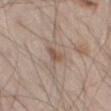Case summary:
- biopsy status — imaged on a skin check; not biopsied
- acquisition — 15 mm crop, total-body photography
- location — the left thigh
- lighting — white-light illumination
- TBP lesion metrics — a border-irregularity index near 3.5/10, a color-variation rating of about 2.5/10, and radial color variation of about 0.5
- patient — male, aged 58–62